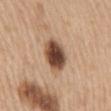Assessment: Recorded during total-body skin imaging; not selected for excision or biopsy. Context: Located on the mid back. A female subject approximately 70 years of age. Imaged with white-light lighting. A close-up tile cropped from a whole-body skin photograph, about 15 mm across. Approximately 4.5 mm at its widest.